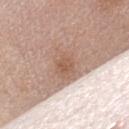Assessment: Captured during whole-body skin photography for melanoma surveillance; the lesion was not biopsied. Image and clinical context: Automated tile analysis of the lesion measured a footprint of about 14 mm² and a shape eccentricity near 0.6. The software also gave border irregularity of about 3.5 on a 0–10 scale. The lesion is on the left upper arm. Measured at roughly 5 mm in maximum diameter. A 15 mm crop from a total-body photograph taken for skin-cancer surveillance. A female subject, in their mid- to late 60s.